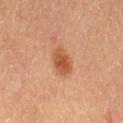Captured during whole-body skin photography for melanoma surveillance; the lesion was not biopsied. A close-up tile cropped from a whole-body skin photograph, about 15 mm across. A female subject, in their 60s. From the left thigh.